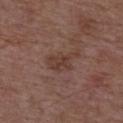location: the upper back
image source: 15 mm crop, total-body photography
illumination: white-light illumination
subject: male, about 50 years old
diameter: ≈4 mm
automated metrics: a lesion color around L≈39 a*≈19 b*≈24 in CIELAB, roughly 6 lightness units darker than nearby skin, and a normalized border contrast of about 6; a border-irregularity index near 5.5/10, a within-lesion color-variation index near 2.5/10, and radial color variation of about 1